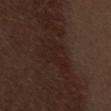Part of a total-body skin-imaging series; this lesion was reviewed on a skin check and was not flagged for biopsy.
Imaged with white-light lighting.
The subject is a male aged approximately 70.
The total-body-photography lesion software estimated an average lesion color of about L≈20 a*≈16 b*≈19 (CIELAB), a lesion–skin lightness drop of about 4, and a lesion-to-skin contrast of about 5.5 (normalized; higher = more distinct). The software also gave a classifier nevus-likeness of about 0/100 and lesion-presence confidence of about 55/100.
A region of skin cropped from a whole-body photographic capture, roughly 15 mm wide.
The lesion is on the abdomen.